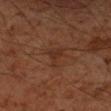Image and clinical context: This image is a 15 mm lesion crop taken from a total-body photograph. The lesion is on the right forearm. The patient is a male roughly 60 years of age. Automated image analysis of the tile measured an area of roughly 4 mm², an outline eccentricity of about 0.7 (0 = round, 1 = elongated), and a symmetry-axis asymmetry near 0.6. It also reported roughly 5 lightness units darker than nearby skin and a normalized lesion–skin contrast near 5.5. And it measured a nevus-likeness score of about 0/100 and lesion-presence confidence of about 100/100. The recorded lesion diameter is about 3 mm. This is a cross-polarized tile.